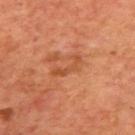The lesion was photographed on a routine skin check and not biopsied; there is no pathology result. A male subject aged 68–72. The lesion is located on the upper back. Cropped from a whole-body photographic skin survey; the tile spans about 15 mm.